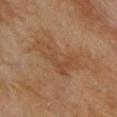{
  "biopsy_status": "not biopsied; imaged during a skin examination",
  "patient": {
    "sex": "female",
    "age_approx": 80
  },
  "lighting": "cross-polarized",
  "automated_metrics": {
    "area_mm2_approx": 14.0,
    "eccentricity": 0.9,
    "shape_asymmetry": 0.5,
    "nevus_likeness_0_100": 0,
    "lesion_detection_confidence_0_100": 100
  },
  "lesion_size": {
    "long_diameter_mm_approx": 6.5
  },
  "image": {
    "source": "total-body photography crop",
    "field_of_view_mm": 15
  },
  "site": "upper back"
}